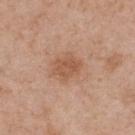Captured under white-light illumination. About 3.5 mm across. On the upper back. A male subject aged approximately 55. A 15 mm close-up tile from a total-body photography series done for melanoma screening.